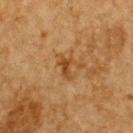  biopsy_status: not biopsied; imaged during a skin examination
  automated_metrics:
    eccentricity: 0.8
    shape_asymmetry: 0.4
    border_irregularity_0_10: 4.5
    peripheral_color_asymmetry: 0.5
  lesion_size:
    long_diameter_mm_approx: 2.5
  lighting: cross-polarized
  patient:
    sex: male
    age_approx: 85+
  image:
    source: total-body photography crop
    field_of_view_mm: 15
  site: back